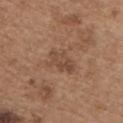biopsy_status: not biopsied; imaged during a skin examination
site: chest
patient:
  sex: male
  age_approx: 65
image:
  source: total-body photography crop
  field_of_view_mm: 15
lesion_size:
  long_diameter_mm_approx: 3.5
lighting: white-light
automated_metrics:
  cielab_L: 47
  cielab_a: 19
  cielab_b: 29
  vs_skin_darker_L: 7.0
  vs_skin_contrast_norm: 5.5
  border_irregularity_0_10: 4.0
  color_variation_0_10: 4.0
  peripheral_color_asymmetry: 1.5